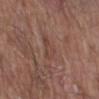Clinical impression: This lesion was catalogued during total-body skin photography and was not selected for biopsy. Context: Approximately 3.5 mm at its widest. Automated image analysis of the tile measured a lesion area of about 6.5 mm², a shape eccentricity near 0.75, and a symmetry-axis asymmetry near 0.35. The software also gave a border-irregularity index near 4/10, a within-lesion color-variation index near 2.5/10, and radial color variation of about 0.5. The analysis additionally found a lesion-detection confidence of about 70/100. A 15 mm close-up tile from a total-body photography series done for melanoma screening. This is a white-light tile. A male subject, in their 80s. Located on the right lower leg.